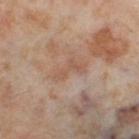Findings:
- biopsy status · imaged on a skin check; not biopsied
- site · the right thigh
- diameter · about 4 mm
- subject · female, roughly 55 years of age
- acquisition · total-body-photography crop, ~15 mm field of view
- illumination · cross-polarized illumination
- image-analysis metrics · a classifier nevus-likeness of about 0/100 and a lesion-detection confidence of about 95/100